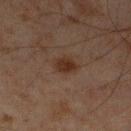Clinical impression:
The lesion was tiled from a total-body skin photograph and was not biopsied.
Background:
A male subject about 45 years old. About 3 mm across. Located on the left lower leg. The tile uses cross-polarized illumination. A lesion tile, about 15 mm wide, cut from a 3D total-body photograph.